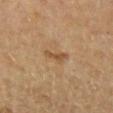Part of a total-body skin-imaging series; this lesion was reviewed on a skin check and was not flagged for biopsy. A 15 mm close-up tile from a total-body photography series done for melanoma screening. The patient is a male aged around 65. From the arm.